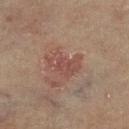workup: no biopsy performed (imaged during a skin exam)
lighting: cross-polarized
image source: 15 mm crop, total-body photography
site: the left lower leg
image-analysis metrics: a lesion color around L≈40 a*≈19 b*≈21 in CIELAB, about 6 CIELAB-L* units darker than the surrounding skin, and a lesion-to-skin contrast of about 5.5 (normalized; higher = more distinct); a border-irregularity index near 4.5/10 and a within-lesion color-variation index near 2/10; a nevus-likeness score of about 0/100 and a detector confidence of about 100 out of 100 that the crop contains a lesion
patient: female, approximately 80 years of age
lesion size: ≈4 mm